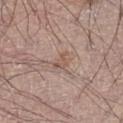Findings:
- follow-up: no biopsy performed (imaged during a skin exam)
- acquisition: ~15 mm tile from a whole-body skin photo
- TBP lesion metrics: a border-irregularity index near 3/10 and a color-variation rating of about 2/10; a nevus-likeness score of about 0/100 and lesion-presence confidence of about 100/100
- patient: male, aged around 65
- location: the right thigh
- size: ~2.5 mm (longest diameter)